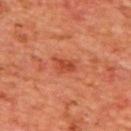<tbp_lesion>
<biopsy_status>not biopsied; imaged during a skin examination</biopsy_status>
<lesion_size>
  <long_diameter_mm_approx>3.0</long_diameter_mm_approx>
</lesion_size>
<patient>
  <sex>male</sex>
  <age_approx>65</age_approx>
</patient>
<site>upper back</site>
<automated_metrics>
  <border_irregularity_0_10>3.5</border_irregularity_0_10>
  <peripheral_color_asymmetry>0.5</peripheral_color_asymmetry>
  <nevus_likeness_0_100>20</nevus_likeness_0_100>
</automated_metrics>
<image>
  <source>total-body photography crop</source>
  <field_of_view_mm>15</field_of_view_mm>
</image>
</tbp_lesion>